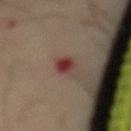biopsy status=no biopsy performed (imaged during a skin exam); tile lighting=cross-polarized illumination; diameter=≈2.5 mm; imaging modality=~15 mm tile from a whole-body skin photo; site=the mid back; patient=male, roughly 50 years of age.